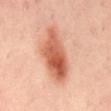The lesion was photographed on a routine skin check and not biopsied; there is no pathology result.
Longest diameter approximately 7.5 mm.
Imaged with cross-polarized lighting.
A male patient, approximately 40 years of age.
A region of skin cropped from a whole-body photographic capture, roughly 15 mm wide.
The lesion is located on the mid back.